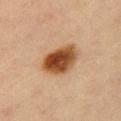The lesion was photographed on a routine skin check and not biopsied; there is no pathology result. An algorithmic analysis of the crop reported a lesion color around L≈42 a*≈20 b*≈32 in CIELAB, about 16 CIELAB-L* units darker than the surrounding skin, and a normalized border contrast of about 12.5. The analysis additionally found border irregularity of about 1.5 on a 0–10 scale and a peripheral color-asymmetry measure near 2.5. Cropped from a total-body skin-imaging series; the visible field is about 15 mm. The subject is a female aged 63–67. The lesion's longest dimension is about 5 mm. Imaged with cross-polarized lighting. The lesion is located on the chest.